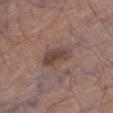Q: Patient demographics?
A: male, approximately 70 years of age
Q: Lesion location?
A: the right thigh
Q: How was this image acquired?
A: total-body-photography crop, ~15 mm field of view